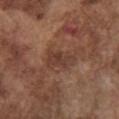The lesion was tiled from a total-body skin photograph and was not biopsied. The lesion is on the chest. Imaged with white-light lighting. The patient is a male aged 73–77. Approximately 4 mm at its widest. Automated tile analysis of the lesion measured border irregularity of about 3.5 on a 0–10 scale and a within-lesion color-variation index near 3/10. It also reported a classifier nevus-likeness of about 0/100 and a lesion-detection confidence of about 100/100. This image is a 15 mm lesion crop taken from a total-body photograph.